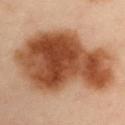Clinical impression: Part of a total-body skin-imaging series; this lesion was reviewed on a skin check and was not flagged for biopsy. Acquisition and patient details: A 15 mm close-up tile from a total-body photography series done for melanoma screening. The total-body-photography lesion software estimated an outline eccentricity of about 0.8 (0 = round, 1 = elongated) and two-axis asymmetry of about 0.3. It also reported a border-irregularity rating of about 3.5/10, a within-lesion color-variation index near 7.5/10, and a peripheral color-asymmetry measure near 2.5. This is a cross-polarized tile. A male subject aged approximately 55. Longest diameter approximately 12.5 mm. Located on the right upper arm.